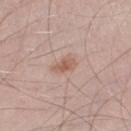This image is a 15 mm lesion crop taken from a total-body photograph. A male subject aged 53–57. The lesion is on the right thigh. Longest diameter approximately 3 mm. Captured under white-light illumination.